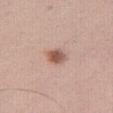{"biopsy_status": "not biopsied; imaged during a skin examination"}